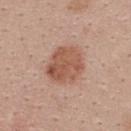<record>
<biopsy_status>not biopsied; imaged during a skin examination</biopsy_status>
<image>
  <source>total-body photography crop</source>
  <field_of_view_mm>15</field_of_view_mm>
</image>
<lesion_size>
  <long_diameter_mm_approx>5.0</long_diameter_mm_approx>
</lesion_size>
<patient>
  <sex>female</sex>
  <age_approx>25</age_approx>
</patient>
<site>upper back</site>
</record>